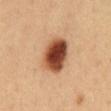Recorded during total-body skin imaging; not selected for excision or biopsy. A male subject about 50 years old. A region of skin cropped from a whole-body photographic capture, roughly 15 mm wide. The lesion is on the front of the torso. An algorithmic analysis of the crop reported a mean CIELAB color near L≈47 a*≈25 b*≈34 and a lesion–skin lightness drop of about 21. And it measured border irregularity of about 1.5 on a 0–10 scale and internal color variation of about 8.5 on a 0–10 scale. It also reported a lesion-detection confidence of about 100/100. Measured at roughly 4.5 mm in maximum diameter.